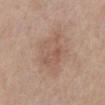{"biopsy_status": "not biopsied; imaged during a skin examination", "patient": {"sex": "female", "age_approx": 65}, "image": {"source": "total-body photography crop", "field_of_view_mm": 15}, "lesion_size": {"long_diameter_mm_approx": 6.5}, "site": "abdomen"}